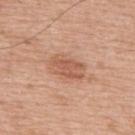Assessment:
The lesion was tiled from a total-body skin photograph and was not biopsied.
Image and clinical context:
A male patient, aged around 55. About 4 mm across. Captured under white-light illumination. A 15 mm close-up tile from a total-body photography series done for melanoma screening. From the upper back.